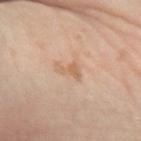| field | value |
|---|---|
| workup | total-body-photography surveillance lesion; no biopsy |